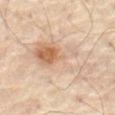notes=imaged on a skin check; not biopsied
patient=male, aged approximately 65
tile lighting=cross-polarized
imaging modality=15 mm crop, total-body photography
site=the abdomen
image-analysis metrics=an average lesion color of about L≈65 a*≈15 b*≈30 (CIELAB), about 8 CIELAB-L* units darker than the surrounding skin, and a normalized border contrast of about 5.5; a border-irregularity index near 7/10, internal color variation of about 9.5 on a 0–10 scale, and radial color variation of about 3